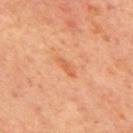The lesion was tiled from a total-body skin photograph and was not biopsied. Located on the back. A 15 mm crop from a total-body photograph taken for skin-cancer surveillance. A male subject in their 70s.